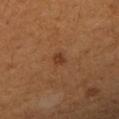{
  "biopsy_status": "not biopsied; imaged during a skin examination",
  "image": {
    "source": "total-body photography crop",
    "field_of_view_mm": 15
  },
  "patient": {
    "sex": "female",
    "age_approx": 55
  },
  "lighting": "cross-polarized",
  "lesion_size": {
    "long_diameter_mm_approx": 1.5
  },
  "site": "arm"
}